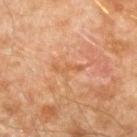Impression:
This lesion was catalogued during total-body skin photography and was not selected for biopsy.
Context:
Automated tile analysis of the lesion measured an area of roughly 2 mm² and a shape eccentricity near 0.95. The software also gave a classifier nevus-likeness of about 0/100 and lesion-presence confidence of about 100/100. This image is a 15 mm lesion crop taken from a total-body photograph. The tile uses cross-polarized illumination. The lesion is on the right forearm. A male subject in their mid-40s.